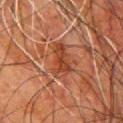Q: Was this lesion biopsied?
A: total-body-photography surveillance lesion; no biopsy
Q: Where on the body is the lesion?
A: the chest
Q: How was this image acquired?
A: 15 mm crop, total-body photography
Q: Automated lesion metrics?
A: a lesion color around L≈34 a*≈23 b*≈29 in CIELAB and a lesion–skin lightness drop of about 7; border irregularity of about 3.5 on a 0–10 scale, internal color variation of about 4 on a 0–10 scale, and peripheral color asymmetry of about 1.5
Q: What are the patient's age and sex?
A: male, approximately 80 years of age
Q: What lighting was used for the tile?
A: cross-polarized illumination